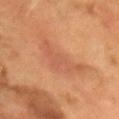Part of a total-body skin-imaging series; this lesion was reviewed on a skin check and was not flagged for biopsy. From the head or neck. The tile uses cross-polarized illumination. The lesion's longest dimension is about 6.5 mm. Cropped from a whole-body photographic skin survey; the tile spans about 15 mm. An algorithmic analysis of the crop reported about 6 CIELAB-L* units darker than the surrounding skin and a normalized lesion–skin contrast near 4.5. And it measured a border-irregularity index near 6.5/10 and peripheral color asymmetry of about 1. It also reported lesion-presence confidence of about 100/100. A male patient about 55 years old.